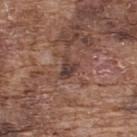{"biopsy_status": "not biopsied; imaged during a skin examination"}